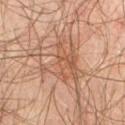Case summary:
• biopsy status: imaged on a skin check; not biopsied
• anatomic site: the chest
• patient: male, aged 63 to 67
• image: ~15 mm tile from a whole-body skin photo